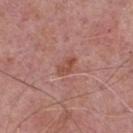This lesion was catalogued during total-body skin photography and was not selected for biopsy. A lesion tile, about 15 mm wide, cut from a 3D total-body photograph. The lesion is on the chest. About 2.5 mm across. A male patient about 75 years old.